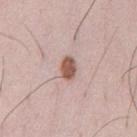Notes:
* workup — imaged on a skin check; not biopsied
* lesion diameter — about 3 mm
* acquisition — 15 mm crop, total-body photography
* subject — male, aged 33–37
* location — the chest
* automated metrics — a border-irregularity rating of about 2/10, a within-lesion color-variation index near 2/10, and radial color variation of about 0.5; a detector confidence of about 100 out of 100 that the crop contains a lesion
* tile lighting — white-light